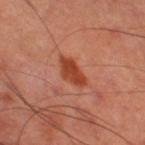Case summary:
• workup · no biopsy performed (imaged during a skin exam)
• patient · male, aged 63 to 67
• body site · the right thigh
• image · 15 mm crop, total-body photography
• illumination · cross-polarized illumination
• size · ≈4 mm